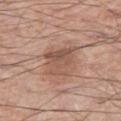Case summary:
– biopsy status: catalogued during a skin exam; not biopsied
– image-analysis metrics: an area of roughly 11 mm², an outline eccentricity of about 0.7 (0 = round, 1 = elongated), and a shape-asymmetry score of about 0.35 (0 = symmetric); a border-irregularity rating of about 4/10, internal color variation of about 5 on a 0–10 scale, and a peripheral color-asymmetry measure near 2
– patient: male, about 60 years old
– imaging modality: 15 mm crop, total-body photography
– lighting: white-light illumination
– size: ≈4 mm
– body site: the right thigh